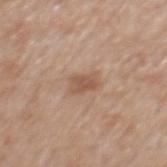The lesion was photographed on a routine skin check and not biopsied; there is no pathology result. A male subject, approximately 65 years of age. The lesion is located on the mid back. This image is a 15 mm lesion crop taken from a total-body photograph.